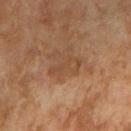workup: imaged on a skin check; not biopsied
subject: female, aged 58–62
site: the arm
imaging modality: ~15 mm tile from a whole-body skin photo
illumination: cross-polarized illumination